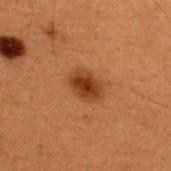  biopsy_status: not biopsied; imaged during a skin examination
  site: upper back
  lighting: cross-polarized
  patient:
    sex: male
    age_approx: 50
  image:
    source: total-body photography crop
    field_of_view_mm: 15